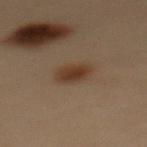{
  "biopsy_status": "not biopsied; imaged during a skin examination"
}